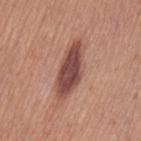| field | value |
|---|---|
| notes | imaged on a skin check; not biopsied |
| subject | female, aged approximately 40 |
| TBP lesion metrics | border irregularity of about 3 on a 0–10 scale, a color-variation rating of about 3.5/10, and peripheral color asymmetry of about 1 |
| illumination | white-light illumination |
| size | ≈7.5 mm |
| site | the left thigh |
| image | 15 mm crop, total-body photography |